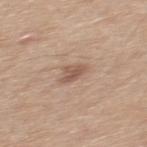{"biopsy_status": "not biopsied; imaged during a skin examination", "automated_metrics": {"cielab_L": 55, "cielab_a": 18, "cielab_b": 27, "vs_skin_darker_L": 10.0, "vs_skin_contrast_norm": 7.0}, "lighting": "white-light", "image": {"source": "total-body photography crop", "field_of_view_mm": 15}, "lesion_size": {"long_diameter_mm_approx": 2.5}, "site": "upper back", "patient": {"sex": "male", "age_approx": 30}}